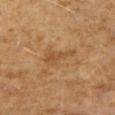- biopsy status · no biopsy performed (imaged during a skin exam)
- size · ~4 mm (longest diameter)
- location · the right upper arm
- patient · female, in their 60s
- image source · ~15 mm crop, total-body skin-cancer survey